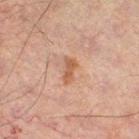<lesion>
<patient>
  <sex>male</sex>
  <age_approx>60</age_approx>
</patient>
<lighting>cross-polarized</lighting>
<site>left leg</site>
<lesion_size>
  <long_diameter_mm_approx>3.0</long_diameter_mm_approx>
</lesion_size>
<image>
  <source>total-body photography crop</source>
  <field_of_view_mm>15</field_of_view_mm>
</image>
</lesion>